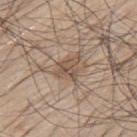notes = catalogued during a skin exam; not biopsied
lesion diameter = about 3.5 mm
acquisition = 15 mm crop, total-body photography
anatomic site = the mid back
patient = male, aged 68–72